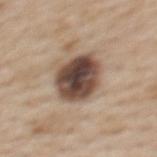No biopsy was performed on this lesion — it was imaged during a full skin examination and was not determined to be concerning. Longest diameter approximately 5 mm. A female patient, aged 48–52. Located on the upper back. Automated tile analysis of the lesion measured internal color variation of about 8.5 on a 0–10 scale and a peripheral color-asymmetry measure near 2.5. The software also gave a detector confidence of about 100 out of 100 that the crop contains a lesion. This is a white-light tile. A 15 mm crop from a total-body photograph taken for skin-cancer surveillance.